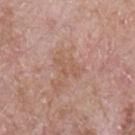Clinical impression: The lesion was tiled from a total-body skin photograph and was not biopsied. Image and clinical context: A male subject in their mid- to late 60s. About 3 mm across. The lesion is located on the right upper arm. This is a white-light tile. This image is a 15 mm lesion crop taken from a total-body photograph.